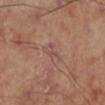Impression:
The lesion was tiled from a total-body skin photograph and was not biopsied.
Background:
On the right lower leg. The tile uses cross-polarized illumination. A 15 mm close-up tile from a total-body photography series done for melanoma screening.